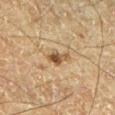Imaged during a routine full-body skin examination; the lesion was not biopsied and no histopathology is available. The patient is a male aged 63–67. A close-up tile cropped from a whole-body skin photograph, about 15 mm across. Captured under cross-polarized illumination. On the leg.